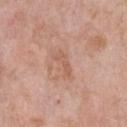This lesion was catalogued during total-body skin photography and was not selected for biopsy. A male patient roughly 50 years of age. On the chest. Captured under white-light illumination. An algorithmic analysis of the crop reported about 7 CIELAB-L* units darker than the surrounding skin and a normalized border contrast of about 5.5. And it measured border irregularity of about 6 on a 0–10 scale, internal color variation of about 0 on a 0–10 scale, and a peripheral color-asymmetry measure near 0. The analysis additionally found a classifier nevus-likeness of about 0/100. A 15 mm close-up tile from a total-body photography series done for melanoma screening. About 3 mm across.